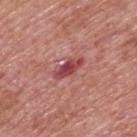Image and clinical context:
Located on the upper back. The recorded lesion diameter is about 4 mm. The tile uses white-light illumination. Cropped from a whole-body photographic skin survey; the tile spans about 15 mm. Automated image analysis of the tile measured a border-irregularity index near 3/10 and radial color variation of about 1.5. A male subject, approximately 75 years of age.